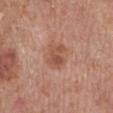No biopsy was performed on this lesion — it was imaged during a full skin examination and was not determined to be concerning. The patient is a male aged 68–72. Imaged with white-light lighting. From the left lower leg. A lesion tile, about 15 mm wide, cut from a 3D total-body photograph. About 3 mm across.